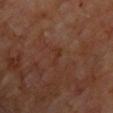{
  "biopsy_status": "not biopsied; imaged during a skin examination"
}